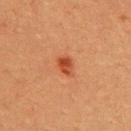lighting = cross-polarized
anatomic site = the upper back
subject = male, approximately 40 years of age
imaging modality = ~15 mm tile from a whole-body skin photo
image-analysis metrics = an eccentricity of roughly 0.75 and a symmetry-axis asymmetry near 0.25; border irregularity of about 2 on a 0–10 scale and a color-variation rating of about 4/10